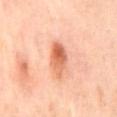workup — catalogued during a skin exam; not biopsied | anatomic site — the mid back | lighting — cross-polarized | subject — female, in their 50s | image source — ~15 mm crop, total-body skin-cancer survey.